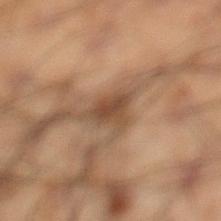Impression:
Recorded during total-body skin imaging; not selected for excision or biopsy.
Context:
On the left lower leg. A region of skin cropped from a whole-body photographic capture, roughly 15 mm wide. The subject is a male aged around 50.